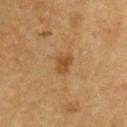Context:
Located on the chest. The subject is a male aged around 60. A region of skin cropped from a whole-body photographic capture, roughly 15 mm wide. Measured at roughly 2.5 mm in maximum diameter. The total-body-photography lesion software estimated a normalized border contrast of about 7.